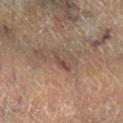follow-up: imaged on a skin check; not biopsied
subject: male, in their mid- to late 80s or older
illumination: cross-polarized
size: ~3 mm (longest diameter)
body site: the right lower leg
image source: ~15 mm crop, total-body skin-cancer survey